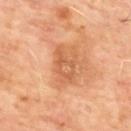Clinical impression: Captured during whole-body skin photography for melanoma surveillance; the lesion was not biopsied. Image and clinical context: The lesion-visualizer software estimated a lesion–skin lightness drop of about 8. And it measured border irregularity of about 4.5 on a 0–10 scale and a color-variation rating of about 4.5/10. And it measured an automated nevus-likeness rating near 0 out of 100 and a lesion-detection confidence of about 100/100. The lesion's longest dimension is about 4 mm. Cropped from a whole-body photographic skin survey; the tile spans about 15 mm. A male patient, in their mid- to late 60s. On the upper back.